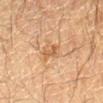Acquisition and patient details: Cropped from a whole-body photographic skin survey; the tile spans about 15 mm. Captured under cross-polarized illumination. A male patient, in their mid-60s. The lesion is located on the left forearm. The total-body-photography lesion software estimated a footprint of about 3 mm². The lesion's longest dimension is about 3 mm.